Captured under white-light illumination. Longest diameter approximately 9 mm. An algorithmic analysis of the crop reported a footprint of about 50 mm², an outline eccentricity of about 0.6 (0 = round, 1 = elongated), and a shape-asymmetry score of about 0.1 (0 = symmetric). And it measured roughly 16 lightness units darker than nearby skin and a lesion-to-skin contrast of about 14.5 (normalized; higher = more distinct). The analysis additionally found an automated nevus-likeness rating near 40 out of 100. The patient is a male in their mid-50s. A lesion tile, about 15 mm wide, cut from a 3D total-body photograph. The lesion is on the chest:
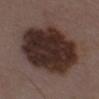Conclusion:
On excision, pathology confirmed a melanoma in situ, lentigo maligna type.